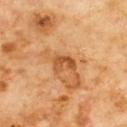<case>
  <biopsy_status>not biopsied; imaged during a skin examination</biopsy_status>
  <lighting>cross-polarized</lighting>
  <image>
    <source>total-body photography crop</source>
    <field_of_view_mm>15</field_of_view_mm>
  </image>
  <lesion_size>
    <long_diameter_mm_approx>3.0</long_diameter_mm_approx>
  </lesion_size>
  <site>chest</site>
  <patient>
    <sex>male</sex>
    <age_approx>60</age_approx>
  </patient>
</case>